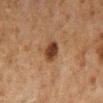The lesion was tiled from a total-body skin photograph and was not biopsied. The lesion's longest dimension is about 2.5 mm. The tile uses cross-polarized illumination. Automated image analysis of the tile measured a footprint of about 5 mm². The software also gave a lesion color around L≈32 a*≈18 b*≈26 in CIELAB, roughly 12 lightness units darker than nearby skin, and a lesion-to-skin contrast of about 11 (normalized; higher = more distinct). And it measured a border-irregularity rating of about 2/10, a color-variation rating of about 2.5/10, and a peripheral color-asymmetry measure near 1. The analysis additionally found a classifier nevus-likeness of about 95/100 and lesion-presence confidence of about 100/100. The lesion is located on the leg. A 15 mm close-up extracted from a 3D total-body photography capture. A male subject aged around 65.